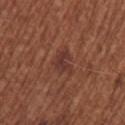Clinical impression:
Recorded during total-body skin imaging; not selected for excision or biopsy.
Image and clinical context:
A female subject, in their mid- to late 60s. The lesion is on the upper back. This image is a 15 mm lesion crop taken from a total-body photograph. Automated tile analysis of the lesion measured a mean CIELAB color near L≈35 a*≈22 b*≈24, a lesion–skin lightness drop of about 7, and a normalized lesion–skin contrast near 7.5. The analysis additionally found a border-irregularity rating of about 4/10, a within-lesion color-variation index near 1/10, and radial color variation of about 0.5. It also reported an automated nevus-likeness rating near 10 out of 100. Imaged with white-light lighting.